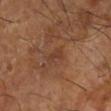biopsy_status: not biopsied; imaged during a skin examination